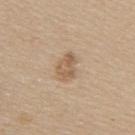Findings:
– workup: no biopsy performed (imaged during a skin exam)
– lesion diameter: about 3.5 mm
– imaging modality: ~15 mm tile from a whole-body skin photo
– TBP lesion metrics: a footprint of about 6 mm², a shape eccentricity near 0.75, and two-axis asymmetry of about 0.25; a lesion color around L≈58 a*≈16 b*≈32 in CIELAB, roughly 9 lightness units darker than nearby skin, and a normalized lesion–skin contrast near 6.5; a border-irregularity rating of about 2.5/10, a within-lesion color-variation index near 3.5/10, and peripheral color asymmetry of about 1; an automated nevus-likeness rating near 20 out of 100 and a detector confidence of about 100 out of 100 that the crop contains a lesion
– lighting: white-light
– site: the right upper arm
– subject: female, in their mid-40s